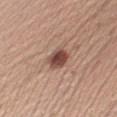| feature | finding |
|---|---|
| workup | total-body-photography surveillance lesion; no biopsy |
| location | the right upper arm |
| image-analysis metrics | a mean CIELAB color near L≈47 a*≈21 b*≈25 and a normalized lesion–skin contrast near 10.5 |
| patient | male, about 35 years old |
| imaging modality | ~15 mm crop, total-body skin-cancer survey |
| diameter | ≈3 mm |
| tile lighting | white-light |